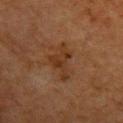Findings:
• workup · no biopsy performed (imaged during a skin exam)
• patient · female, roughly 55 years of age
• body site · the upper back
• image · 15 mm crop, total-body photography